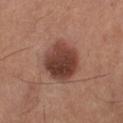The lesion was tiled from a total-body skin photograph and was not biopsied. The tile uses cross-polarized illumination. Located on the left lower leg. The lesion's longest dimension is about 6 mm. A 15 mm crop from a total-body photograph taken for skin-cancer surveillance. Automated image analysis of the tile measured a border-irregularity index near 2/10, a color-variation rating of about 6/10, and radial color variation of about 2. It also reported a lesion-detection confidence of about 100/100. A female subject aged 58–62.